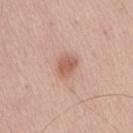This lesion was catalogued during total-body skin photography and was not selected for biopsy. A lesion tile, about 15 mm wide, cut from a 3D total-body photograph. A male subject, roughly 55 years of age. About 3 mm across. The lesion is on the lower back. Imaged with white-light lighting.